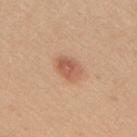follow-up: no biopsy performed (imaged during a skin exam) | anatomic site: the back | automated lesion analysis: an eccentricity of roughly 0.75 and two-axis asymmetry of about 0.2; a lesion color around L≈57 a*≈24 b*≈32 in CIELAB, about 11 CIELAB-L* units darker than the surrounding skin, and a lesion-to-skin contrast of about 7 (normalized; higher = more distinct) | image: ~15 mm tile from a whole-body skin photo | diameter: ≈3 mm | tile lighting: white-light illumination | patient: female, aged around 40.